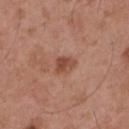Context: A 15 mm close-up extracted from a 3D total-body photography capture. The lesion's longest dimension is about 2.5 mm. A male subject, aged 53–57. Located on the mid back. The tile uses white-light illumination.